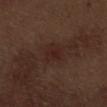biopsy status — imaged on a skin check; not biopsied | acquisition — 15 mm crop, total-body photography | lesion diameter — about 3 mm | anatomic site — the abdomen | illumination — white-light | patient — male, about 70 years old.